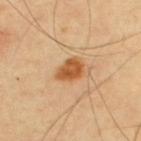subject: male, roughly 65 years of age | acquisition: ~15 mm crop, total-body skin-cancer survey | image-analysis metrics: a footprint of about 6.5 mm², an outline eccentricity of about 0.65 (0 = round, 1 = elongated), and a shape-asymmetry score of about 0.25 (0 = symmetric); an average lesion color of about L≈55 a*≈25 b*≈41 (CIELAB), a lesion–skin lightness drop of about 13, and a lesion-to-skin contrast of about 10 (normalized; higher = more distinct) | diameter: about 3.5 mm | anatomic site: the upper back | tile lighting: cross-polarized.